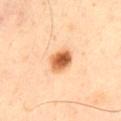Imaged during a routine full-body skin examination; the lesion was not biopsied and no histopathology is available. Located on the left thigh. A male patient about 50 years old. Cropped from a total-body skin-imaging series; the visible field is about 15 mm.